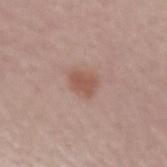The lesion was tiled from a total-body skin photograph and was not biopsied. This image is a 15 mm lesion crop taken from a total-body photograph. Approximately 3 mm at its widest. Captured under white-light illumination. The lesion is located on the left forearm. The subject is a male about 60 years old.